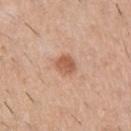subject = male, aged 58 to 62; lesion diameter = about 2.5 mm; imaging modality = ~15 mm crop, total-body skin-cancer survey; location = the right upper arm; illumination = white-light illumination.